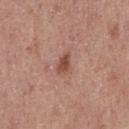Captured during whole-body skin photography for melanoma surveillance; the lesion was not biopsied. The patient is a female aged approximately 40. A 15 mm close-up tile from a total-body photography series done for melanoma screening. About 3 mm across. Captured under white-light illumination. The lesion is on the right thigh.